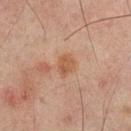Q: Was a biopsy performed?
A: catalogued during a skin exam; not biopsied
Q: What kind of image is this?
A: 15 mm crop, total-body photography
Q: Patient demographics?
A: male, aged approximately 65
Q: Where on the body is the lesion?
A: the mid back
Q: What lighting was used for the tile?
A: cross-polarized
Q: What is the lesion's diameter?
A: ≈3 mm